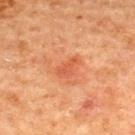Q: What is the lesion's diameter?
A: about 3 mm
Q: What lighting was used for the tile?
A: cross-polarized
Q: Patient demographics?
A: male, aged 63–67
Q: What kind of image is this?
A: ~15 mm tile from a whole-body skin photo
Q: Where on the body is the lesion?
A: the upper back